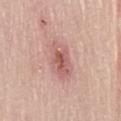Image and clinical context:
The tile uses white-light illumination. A female subject aged approximately 75. Located on the lower back. A 15 mm crop from a total-body photograph taken for skin-cancer surveillance. The lesion's longest dimension is about 5 mm.